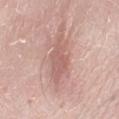Findings:
– workup: imaged on a skin check; not biopsied
– imaging modality: ~15 mm tile from a whole-body skin photo
– lighting: white-light
– subject: male, in their 60s
– image-analysis metrics: an average lesion color of about L≈60 a*≈22 b*≈24 (CIELAB), about 9 CIELAB-L* units darker than the surrounding skin, and a normalized lesion–skin contrast near 6; an automated nevus-likeness rating near 0 out of 100 and lesion-presence confidence of about 100/100
– lesion diameter: about 6 mm
– body site: the leg